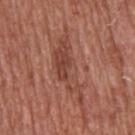<tbp_lesion>
<biopsy_status>not biopsied; imaged during a skin examination</biopsy_status>
<lighting>white-light</lighting>
<site>upper back</site>
<patient>
  <sex>male</sex>
  <age_approx>65</age_approx>
</patient>
<image>
  <source>total-body photography crop</source>
  <field_of_view_mm>15</field_of_view_mm>
</image>
</tbp_lesion>